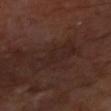Assessment: The lesion was tiled from a total-body skin photograph and was not biopsied. Clinical summary: Cropped from a whole-body photographic skin survey; the tile spans about 15 mm. Automated image analysis of the tile measured a shape eccentricity near 0.9 and a symmetry-axis asymmetry near 0.25. And it measured a border-irregularity index near 4.5/10, a color-variation rating of about 3/10, and a peripheral color-asymmetry measure near 1. Located on the left forearm. The patient is a male approximately 65 years of age. Imaged with cross-polarized lighting. Longest diameter approximately 5 mm.